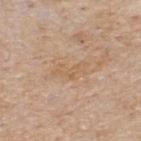Q: Is there a histopathology result?
A: catalogued during a skin exam; not biopsied
Q: How large is the lesion?
A: about 4 mm
Q: Lesion location?
A: the upper back
Q: What did automated image analysis measure?
A: an average lesion color of about L≈60 a*≈17 b*≈34 (CIELAB) and a lesion-to-skin contrast of about 4.5 (normalized; higher = more distinct); a color-variation rating of about 0/10
Q: What are the patient's age and sex?
A: male, aged approximately 55
Q: Illumination type?
A: white-light illumination
Q: What kind of image is this?
A: ~15 mm tile from a whole-body skin photo